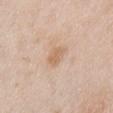Imaged during a routine full-body skin examination; the lesion was not biopsied and no histopathology is available. The lesion is on the abdomen. A male subject aged 63 to 67. Longest diameter approximately 3 mm. A 15 mm close-up tile from a total-body photography series done for melanoma screening. This is a white-light tile.